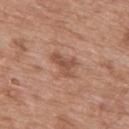• body site: the mid back
• image: 15 mm crop, total-body photography
• lesion size: ≈3.5 mm
• lighting: white-light
• patient: male, aged 48–52